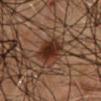Captured during whole-body skin photography for melanoma surveillance; the lesion was not biopsied. Longest diameter approximately 4 mm. A male subject, aged approximately 50. The total-body-photography lesion software estimated an area of roughly 9 mm², a shape eccentricity near 0.75, and a symmetry-axis asymmetry near 0.15. It also reported about 10 CIELAB-L* units darker than the surrounding skin and a normalized border contrast of about 11.5. The software also gave a border-irregularity rating of about 1.5/10 and a within-lesion color-variation index near 3/10. It also reported a classifier nevus-likeness of about 95/100 and lesion-presence confidence of about 100/100. On the mid back. This image is a 15 mm lesion crop taken from a total-body photograph.